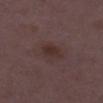follow-up: catalogued during a skin exam; not biopsied
location: the left thigh
automated lesion analysis: a footprint of about 5 mm², a shape eccentricity near 0.8, and a shape-asymmetry score of about 0.2 (0 = symmetric); a mean CIELAB color near L≈30 a*≈17 b*≈17, a lesion–skin lightness drop of about 6, and a normalized border contrast of about 7; an automated nevus-likeness rating near 15 out of 100 and a detector confidence of about 100 out of 100 that the crop contains a lesion
diameter: ≈3 mm
lighting: white-light illumination
imaging modality: ~15 mm tile from a whole-body skin photo
subject: female, about 30 years old